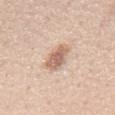| field | value |
|---|---|
| workup | imaged on a skin check; not biopsied |
| body site | the chest |
| illumination | white-light illumination |
| lesion diameter | about 4 mm |
| acquisition | 15 mm crop, total-body photography |
| automated lesion analysis | border irregularity of about 2.5 on a 0–10 scale, internal color variation of about 4 on a 0–10 scale, and radial color variation of about 1; a nevus-likeness score of about 90/100 and a lesion-detection confidence of about 100/100 |
| patient | male, aged 38 to 42 |